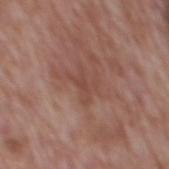Imaged during a routine full-body skin examination; the lesion was not biopsied and no histopathology is available.
The total-body-photography lesion software estimated a mean CIELAB color near L≈46 a*≈22 b*≈25, about 6 CIELAB-L* units darker than the surrounding skin, and a normalized lesion–skin contrast near 5. The analysis additionally found a border-irregularity rating of about 9.5/10, a color-variation rating of about 0/10, and peripheral color asymmetry of about 0. And it measured an automated nevus-likeness rating near 0 out of 100 and lesion-presence confidence of about 90/100.
From the mid back.
A male subject, aged approximately 70.
Cropped from a whole-body photographic skin survey; the tile spans about 15 mm.
Captured under white-light illumination.